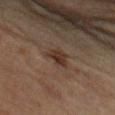This lesion was catalogued during total-body skin photography and was not selected for biopsy.
The recorded lesion diameter is about 3.5 mm.
Automated tile analysis of the lesion measured a footprint of about 5.5 mm², an outline eccentricity of about 0.75 (0 = round, 1 = elongated), and a symmetry-axis asymmetry near 0.35. The software also gave an average lesion color of about L≈28 a*≈15 b*≈22 (CIELAB) and a lesion-to-skin contrast of about 8 (normalized; higher = more distinct). The analysis additionally found a border-irregularity index near 3.5/10 and peripheral color asymmetry of about 1. The analysis additionally found an automated nevus-likeness rating near 70 out of 100 and a lesion-detection confidence of about 100/100.
A 15 mm crop from a total-body photograph taken for skin-cancer surveillance.
On the left forearm.
A female patient about 65 years old.